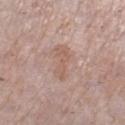Part of a total-body skin-imaging series; this lesion was reviewed on a skin check and was not flagged for biopsy. Automated tile analysis of the lesion measured an area of roughly 6.5 mm², an eccentricity of roughly 0.9, and a symmetry-axis asymmetry near 0.25. The software also gave about 7 CIELAB-L* units darker than the surrounding skin and a lesion-to-skin contrast of about 5 (normalized; higher = more distinct). And it measured radial color variation of about 1. It also reported a classifier nevus-likeness of about 0/100 and a detector confidence of about 100 out of 100 that the crop contains a lesion. A 15 mm crop from a total-body photograph taken for skin-cancer surveillance. Captured under white-light illumination. The lesion is located on the right lower leg. A female patient, aged approximately 60. Measured at roughly 4 mm in maximum diameter.